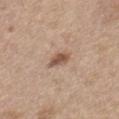The lesion was photographed on a routine skin check and not biopsied; there is no pathology result. The lesion is located on the left thigh. The total-body-photography lesion software estimated border irregularity of about 2.5 on a 0–10 scale, a color-variation rating of about 2.5/10, and peripheral color asymmetry of about 1. Cropped from a whole-body photographic skin survey; the tile spans about 15 mm. This is a white-light tile. About 2.5 mm across. A male patient roughly 70 years of age.